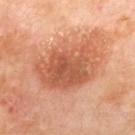The lesion was photographed on a routine skin check and not biopsied; there is no pathology result.
A female patient aged around 50.
An algorithmic analysis of the crop reported a lesion area of about 29 mm², an eccentricity of roughly 0.75, and a shape-asymmetry score of about 0.2 (0 = symmetric). The software also gave a lesion-detection confidence of about 100/100.
Cropped from a total-body skin-imaging series; the visible field is about 15 mm.
The lesion is on the upper back.